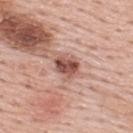Imaged during a routine full-body skin examination; the lesion was not biopsied and no histopathology is available. A male subject approximately 55 years of age. This is a white-light tile. A region of skin cropped from a whole-body photographic capture, roughly 15 mm wide. The lesion is located on the upper back.